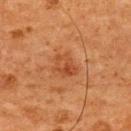Assessment: No biopsy was performed on this lesion — it was imaged during a full skin examination and was not determined to be concerning. Image and clinical context: From the upper back. An algorithmic analysis of the crop reported an area of roughly 6 mm² and a shape eccentricity near 0.7. The analysis additionally found a lesion–skin lightness drop of about 7 and a normalized border contrast of about 5.5. And it measured a border-irregularity index near 3/10, a within-lesion color-variation index near 5.5/10, and radial color variation of about 2. It also reported a lesion-detection confidence of about 100/100. A male patient, aged approximately 65. A region of skin cropped from a whole-body photographic capture, roughly 15 mm wide.